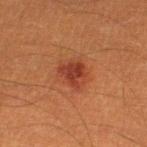notes=catalogued during a skin exam; not biopsied
lesion diameter=~3 mm (longest diameter)
anatomic site=the left lower leg
illumination=cross-polarized
subject=male, aged approximately 40
image=total-body-photography crop, ~15 mm field of view